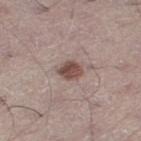notes=no biopsy performed (imaged during a skin exam) | tile lighting=white-light | imaging modality=total-body-photography crop, ~15 mm field of view | subject=male, aged approximately 60 | site=the right thigh.